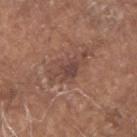biopsy_status: not biopsied; imaged during a skin examination